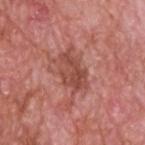<lesion>
<biopsy_status>not biopsied; imaged during a skin examination</biopsy_status>
<site>upper back</site>
<lighting>white-light</lighting>
<image>
  <source>total-body photography crop</source>
  <field_of_view_mm>15</field_of_view_mm>
</image>
<patient>
  <sex>male</sex>
  <age_approx>60</age_approx>
</patient>
<automated_metrics>
  <border_irregularity_0_10>4.0</border_irregularity_0_10>
  <color_variation_0_10>3.5</color_variation_0_10>
  <nevus_likeness_0_100>0</nevus_likeness_0_100>
  <lesion_detection_confidence_0_100>100</lesion_detection_confidence_0_100>
</automated_metrics>
</lesion>